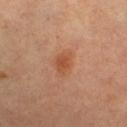Impression: Captured during whole-body skin photography for melanoma surveillance; the lesion was not biopsied. Background: The tile uses cross-polarized illumination. Located on the right lower leg. The recorded lesion diameter is about 3 mm. A 15 mm close-up tile from a total-body photography series done for melanoma screening. A female patient aged 63 to 67.